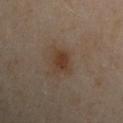<record>
<biopsy_status>not biopsied; imaged during a skin examination</biopsy_status>
<patient>
  <sex>male</sex>
  <age_approx>50</age_approx>
</patient>
<automated_metrics>
  <border_irregularity_0_10>2.5</border_irregularity_0_10>
</automated_metrics>
<site>left upper arm</site>
<lighting>cross-polarized</lighting>
<image>
  <source>total-body photography crop</source>
  <field_of_view_mm>15</field_of_view_mm>
</image>
<lesion_size>
  <long_diameter_mm_approx>3.0</long_diameter_mm_approx>
</lesion_size>
</record>